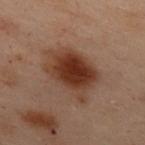Image and clinical context: On the back. Measured at roughly 7 mm in maximum diameter. Imaged with cross-polarized lighting. The subject is a female aged 53 to 57. A roughly 15 mm field-of-view crop from a total-body skin photograph.